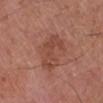Recorded during total-body skin imaging; not selected for excision or biopsy.
The patient is a male aged around 85 or older.
The total-body-photography lesion software estimated an area of roughly 11 mm², a shape eccentricity near 0.8, and a shape-asymmetry score of about 0.4 (0 = symmetric). And it measured a lesion color around L≈46 a*≈24 b*≈28 in CIELAB, about 7 CIELAB-L* units darker than the surrounding skin, and a lesion-to-skin contrast of about 5.5 (normalized; higher = more distinct). It also reported a nevus-likeness score of about 5/100 and a lesion-detection confidence of about 100/100.
This image is a 15 mm lesion crop taken from a total-body photograph.
On the right lower leg.
This is a white-light tile.